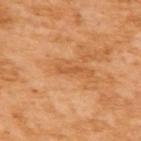notes = no biopsy performed (imaged during a skin exam)
size = ≈2.5 mm
subject = female, aged approximately 55
tile lighting = cross-polarized illumination
automated metrics = a mean CIELAB color near L≈56 a*≈27 b*≈44 and roughly 8 lightness units darker than nearby skin; a color-variation rating of about 0/10 and radial color variation of about 0; a lesion-detection confidence of about 95/100
imaging modality = 15 mm crop, total-body photography
site = the upper back